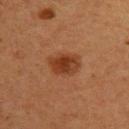A female subject, roughly 40 years of age. A 15 mm close-up tile from a total-body photography series done for melanoma screening. From the upper back. Measured at roughly 4 mm in maximum diameter.